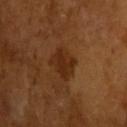The lesion was photographed on a routine skin check and not biopsied; there is no pathology result. A 15 mm close-up extracted from a 3D total-body photography capture. The subject is a male aged around 65. The tile uses cross-polarized illumination. An algorithmic analysis of the crop reported border irregularity of about 2.5 on a 0–10 scale, internal color variation of about 1.5 on a 0–10 scale, and radial color variation of about 0.5. Approximately 3 mm at its widest.